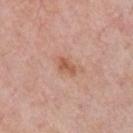This lesion was catalogued during total-body skin photography and was not selected for biopsy. The patient is a male aged 53 to 57. On the chest. This image is a 15 mm lesion crop taken from a total-body photograph. Longest diameter approximately 3 mm.